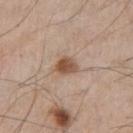{"biopsy_status": "not biopsied; imaged during a skin examination", "site": "left thigh", "image": {"source": "total-body photography crop", "field_of_view_mm": 15}, "patient": {"sex": "male", "age_approx": 60}}